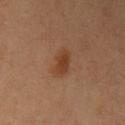Recorded during total-body skin imaging; not selected for excision or biopsy.
A female subject, aged approximately 35.
On the left upper arm.
A 15 mm crop from a total-body photograph taken for skin-cancer surveillance.